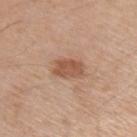Clinical impression:
Imaged during a routine full-body skin examination; the lesion was not biopsied and no histopathology is available.
Acquisition and patient details:
The lesion is located on the arm. A roughly 15 mm field-of-view crop from a total-body skin photograph. This is a white-light tile. Automated image analysis of the tile measured a mean CIELAB color near L≈54 a*≈21 b*≈31, about 11 CIELAB-L* units darker than the surrounding skin, and a normalized border contrast of about 7.5. And it measured border irregularity of about 2 on a 0–10 scale and a within-lesion color-variation index near 3/10. A male subject, roughly 60 years of age. Approximately 3.5 mm at its widest.